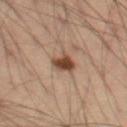Q: Automated lesion metrics?
A: an area of roughly 5 mm² and a shape-asymmetry score of about 0.25 (0 = symmetric); a mean CIELAB color near L≈36 a*≈16 b*≈24 and a normalized border contrast of about 10.5
Q: What is the anatomic site?
A: the left thigh
Q: Patient demographics?
A: male, aged 53–57
Q: What lighting was used for the tile?
A: cross-polarized
Q: What kind of image is this?
A: total-body-photography crop, ~15 mm field of view
Q: What is the lesion's diameter?
A: ≈3 mm